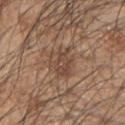Located on the right upper arm. A close-up tile cropped from a whole-body skin photograph, about 15 mm across. The recorded lesion diameter is about 4 mm. A male subject approximately 45 years of age. The total-body-photography lesion software estimated a lesion area of about 8 mm², an outline eccentricity of about 0.7 (0 = round, 1 = elongated), and a symmetry-axis asymmetry near 0.35. And it measured an average lesion color of about L≈46 a*≈18 b*≈27 (CIELAB), roughly 8 lightness units darker than nearby skin, and a normalized lesion–skin contrast near 6.5.